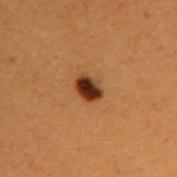<tbp_lesion>
  <lesion_size>
    <long_diameter_mm_approx>3.0</long_diameter_mm_approx>
  </lesion_size>
  <patient>
    <sex>male</sex>
    <age_approx>50</age_approx>
  </patient>
  <site>arm</site>
  <image>
    <source>total-body photography crop</source>
    <field_of_view_mm>15</field_of_view_mm>
  </image>
  <lighting>cross-polarized</lighting>
</tbp_lesion>